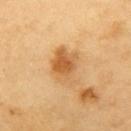Q: Was a biopsy performed?
A: no biopsy performed (imaged during a skin exam)
Q: Illumination type?
A: cross-polarized illumination
Q: What is the anatomic site?
A: the left upper arm
Q: Who is the patient?
A: male, aged around 60
Q: What did automated image analysis measure?
A: an automated nevus-likeness rating near 70 out of 100 and lesion-presence confidence of about 100/100
Q: What is the imaging modality?
A: ~15 mm crop, total-body skin-cancer survey
Q: Lesion size?
A: ~4.5 mm (longest diameter)